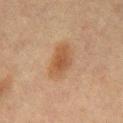workup = catalogued during a skin exam; not biopsied | subject = male, about 65 years old | image = ~15 mm tile from a whole-body skin photo | lesion size = ≈5 mm | body site = the chest.